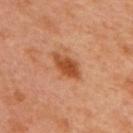Clinical impression:
Imaged during a routine full-body skin examination; the lesion was not biopsied and no histopathology is available.
Background:
A close-up tile cropped from a whole-body skin photograph, about 15 mm across. The recorded lesion diameter is about 4 mm. The tile uses cross-polarized illumination. A male patient, aged approximately 50. The lesion is on the upper back.